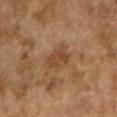This lesion was catalogued during total-body skin photography and was not selected for biopsy. The patient is a female in their 60s. The tile uses cross-polarized illumination. The lesion is on the right upper arm. Cropped from a total-body skin-imaging series; the visible field is about 15 mm. The total-body-photography lesion software estimated a footprint of about 6.5 mm², a shape eccentricity near 0.85, and a shape-asymmetry score of about 0.35 (0 = symmetric). The software also gave a lesion–skin lightness drop of about 7 and a normalized lesion–skin contrast near 6.5. The software also gave a peripheral color-asymmetry measure near 1.